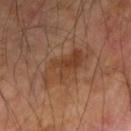notes — no biopsy performed (imaged during a skin exam)
patient — male, roughly 70 years of age
location — the right forearm
image — ~15 mm crop, total-body skin-cancer survey